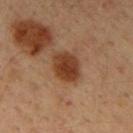Captured during whole-body skin photography for melanoma surveillance; the lesion was not biopsied.